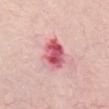workup: no biopsy performed (imaged during a skin exam)
patient: female, approximately 65 years of age
anatomic site: the mid back
image: ~15 mm crop, total-body skin-cancer survey
automated lesion analysis: a mean CIELAB color near L≈58 a*≈38 b*≈20, about 16 CIELAB-L* units darker than the surrounding skin, and a lesion-to-skin contrast of about 10 (normalized; higher = more distinct); a within-lesion color-variation index near 8.5/10 and peripheral color asymmetry of about 2.5; a lesion-detection confidence of about 100/100
lighting: white-light illumination
size: ≈4 mm